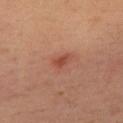{"biopsy_status": "not biopsied; imaged during a skin examination", "automated_metrics": {"eccentricity": 0.8, "shape_asymmetry": 0.35, "cielab_L": 45, "cielab_a": 28, "cielab_b": 30, "vs_skin_darker_L": 9.0, "vs_skin_contrast_norm": 7.0}, "patient": {"sex": "female", "age_approx": 35}, "site": "chest", "image": {"source": "total-body photography crop", "field_of_view_mm": 15}, "lighting": "cross-polarized"}